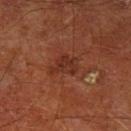A region of skin cropped from a whole-body photographic capture, roughly 15 mm wide. On the left lower leg. A male patient aged 63–67.